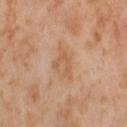<tbp_lesion>
  <biopsy_status>not biopsied; imaged during a skin examination</biopsy_status>
  <automated_metrics>
    <area_mm2_approx>8.5</area_mm2_approx>
    <cielab_L>60</cielab_L>
    <cielab_a>20</cielab_a>
    <cielab_b>35</cielab_b>
    <vs_skin_darker_L>7.0</vs_skin_darker_L>
    <vs_skin_contrast_norm>5.0</vs_skin_contrast_norm>
  </automated_metrics>
  <lesion_size>
    <long_diameter_mm_approx>4.0</long_diameter_mm_approx>
  </lesion_size>
  <site>left thigh</site>
  <image>
    <source>total-body photography crop</source>
    <field_of_view_mm>15</field_of_view_mm>
  </image>
  <patient>
    <sex>female</sex>
    <age_approx>55</age_approx>
  </patient>
</tbp_lesion>